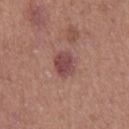Part of a total-body skin-imaging series; this lesion was reviewed on a skin check and was not flagged for biopsy. Located on the leg. A lesion tile, about 15 mm wide, cut from a 3D total-body photograph. The patient is a female roughly 40 years of age. Measured at roughly 3.5 mm in maximum diameter.